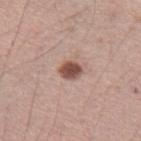Part of a total-body skin-imaging series; this lesion was reviewed on a skin check and was not flagged for biopsy.
A male subject approximately 35 years of age.
A 15 mm close-up tile from a total-body photography series done for melanoma screening.
Captured under white-light illumination.
From the left upper arm.
About 2.5 mm across.